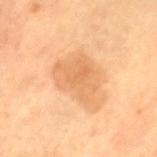Measured at roughly 5.5 mm in maximum diameter.
A female subject, aged 68 to 72.
A close-up tile cropped from a whole-body skin photograph, about 15 mm across.
The lesion is located on the left thigh.